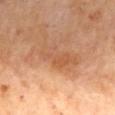Assessment:
This lesion was catalogued during total-body skin photography and was not selected for biopsy.
Image and clinical context:
Located on the mid back. The lesion's longest dimension is about 6 mm. A roughly 15 mm field-of-view crop from a total-body skin photograph. The patient is a male aged 68–72. The tile uses cross-polarized illumination.